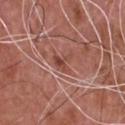Impression:
Captured during whole-body skin photography for melanoma surveillance; the lesion was not biopsied.
Context:
The recorded lesion diameter is about 2.5 mm. A 15 mm close-up tile from a total-body photography series done for melanoma screening. The subject is a male in their mid-70s. The total-body-photography lesion software estimated an average lesion color of about L≈46 a*≈25 b*≈27 (CIELAB), a lesion–skin lightness drop of about 9, and a normalized border contrast of about 7. And it measured border irregularity of about 6.5 on a 0–10 scale and radial color variation of about 0. Captured under white-light illumination. The lesion is on the chest.